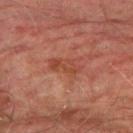The lesion was photographed on a routine skin check and not biopsied; there is no pathology result.
This is a cross-polarized tile.
Longest diameter approximately 4 mm.
A 15 mm close-up extracted from a 3D total-body photography capture.
The lesion is on the leg.
The total-body-photography lesion software estimated an eccentricity of roughly 0.85 and two-axis asymmetry of about 0.35. The analysis additionally found a border-irregularity index near 4/10, internal color variation of about 3 on a 0–10 scale, and peripheral color asymmetry of about 1.
A male patient about 75 years old.